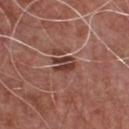The subject is a male aged approximately 55. This image is a 15 mm lesion crop taken from a total-body photograph. An algorithmic analysis of the crop reported a footprint of about 4.5 mm², an outline eccentricity of about 0.7 (0 = round, 1 = elongated), and a shape-asymmetry score of about 0.2 (0 = symmetric). The analysis additionally found a mean CIELAB color near L≈39 a*≈24 b*≈25 and a lesion–skin lightness drop of about 12. And it measured a within-lesion color-variation index near 5/10 and radial color variation of about 2.5. The software also gave a nevus-likeness score of about 0/100 and lesion-presence confidence of about 100/100. On the front of the torso. Imaged with white-light lighting. Approximately 2.5 mm at its widest.